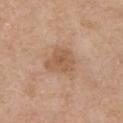<lesion>
  <biopsy_status>not biopsied; imaged during a skin examination</biopsy_status>
  <image>
    <source>total-body photography crop</source>
    <field_of_view_mm>15</field_of_view_mm>
  </image>
  <site>chest</site>
  <lighting>white-light</lighting>
  <lesion_size>
    <long_diameter_mm_approx>4.0</long_diameter_mm_approx>
  </lesion_size>
  <patient>
    <sex>male</sex>
    <age_approx>70</age_approx>
  </patient>
</lesion>